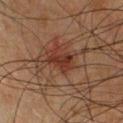Clinical impression:
Imaged during a routine full-body skin examination; the lesion was not biopsied and no histopathology is available.
Background:
A region of skin cropped from a whole-body photographic capture, roughly 15 mm wide. A male patient, aged around 50. The lesion is located on the chest.